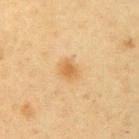Part of a total-body skin-imaging series; this lesion was reviewed on a skin check and was not flagged for biopsy.
On the arm.
A female patient, aged approximately 55.
A close-up tile cropped from a whole-body skin photograph, about 15 mm across.